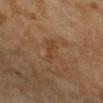Case summary:
* follow-up · imaged on a skin check; not biopsied
* lesion size · ~4 mm (longest diameter)
* TBP lesion metrics · a mean CIELAB color near L≈43 a*≈20 b*≈32, a lesion–skin lightness drop of about 5, and a normalized border contrast of about 4.5; a nevus-likeness score of about 0/100 and a lesion-detection confidence of about 100/100
* tile lighting · cross-polarized
* imaging modality · ~15 mm tile from a whole-body skin photo
* body site · the left forearm
* patient · female, approximately 70 years of age